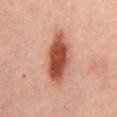Part of a total-body skin-imaging series; this lesion was reviewed on a skin check and was not flagged for biopsy. Automated image analysis of the tile measured an average lesion color of about L≈42 a*≈24 b*≈27 (CIELAB) and a normalized lesion–skin contrast near 11.5. The analysis additionally found a border-irregularity rating of about 2/10 and internal color variation of about 4.5 on a 0–10 scale. A male subject, aged 28 to 32. Cropped from a whole-body photographic skin survey; the tile spans about 15 mm. The lesion is on the back. The tile uses cross-polarized illumination. Longest diameter approximately 7 mm.